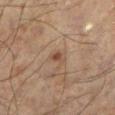The lesion was tiled from a total-body skin photograph and was not biopsied.